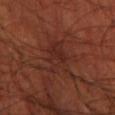Assessment:
This lesion was catalogued during total-body skin photography and was not selected for biopsy.
Background:
A male subject roughly 70 years of age. Automated image analysis of the tile measured a lesion color around L≈26 a*≈22 b*≈23 in CIELAB, about 5 CIELAB-L* units darker than the surrounding skin, and a lesion-to-skin contrast of about 6 (normalized; higher = more distinct). Approximately 5 mm at its widest. From the left thigh. A region of skin cropped from a whole-body photographic capture, roughly 15 mm wide.